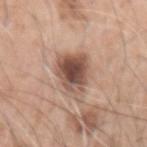Clinical impression: The lesion was photographed on a routine skin check and not biopsied; there is no pathology result. Background: A region of skin cropped from a whole-body photographic capture, roughly 15 mm wide. Imaged with white-light lighting. The patient is a male approximately 60 years of age. Approximately 6 mm at its widest. Automated tile analysis of the lesion measured an automated nevus-likeness rating near 80 out of 100 and a detector confidence of about 100 out of 100 that the crop contains a lesion. On the left upper arm.